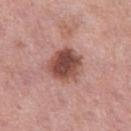Clinical impression: Part of a total-body skin-imaging series; this lesion was reviewed on a skin check and was not flagged for biopsy. Acquisition and patient details: This image is a 15 mm lesion crop taken from a total-body photograph. On the left thigh. A female subject, in their 50s. Captured under white-light illumination. Longest diameter approximately 4 mm.